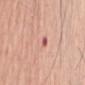{
  "biopsy_status": "not biopsied; imaged during a skin examination",
  "site": "arm",
  "image": {
    "source": "total-body photography crop",
    "field_of_view_mm": 15
  },
  "patient": {
    "sex": "male",
    "age_approx": 55
  }
}